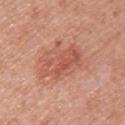No biopsy was performed on this lesion — it was imaged during a full skin examination and was not determined to be concerning.
A region of skin cropped from a whole-body photographic capture, roughly 15 mm wide.
A female subject roughly 50 years of age.
This is a white-light tile.
Located on the arm.
The lesion's longest dimension is about 6 mm.
An algorithmic analysis of the crop reported a mean CIELAB color near L≈55 a*≈28 b*≈30, about 9 CIELAB-L* units darker than the surrounding skin, and a normalized lesion–skin contrast near 6. The analysis additionally found a border-irregularity index near 3.5/10 and internal color variation of about 5 on a 0–10 scale.